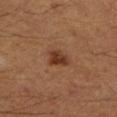A 15 mm close-up extracted from a 3D total-body photography capture.
A male subject, approximately 60 years of age.
The lesion is on the left lower leg.